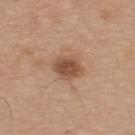Imaged during a routine full-body skin examination; the lesion was not biopsied and no histopathology is available. Located on the upper back. This image is a 15 mm lesion crop taken from a total-body photograph. The tile uses white-light illumination. The lesion-visualizer software estimated a lesion area of about 7.5 mm² and a symmetry-axis asymmetry near 0.15. It also reported a nevus-likeness score of about 85/100. The lesion's longest dimension is about 3.5 mm. The subject is a male in their mid- to late 30s.